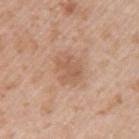Assessment:
Part of a total-body skin-imaging series; this lesion was reviewed on a skin check and was not flagged for biopsy.
Background:
On the left upper arm. Longest diameter approximately 3 mm. A male subject aged 48 to 52. A roughly 15 mm field-of-view crop from a total-body skin photograph.